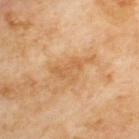The lesion was tiled from a total-body skin photograph and was not biopsied.
The tile uses cross-polarized illumination.
The lesion is on the upper back.
A male patient roughly 70 years of age.
A lesion tile, about 15 mm wide, cut from a 3D total-body photograph.